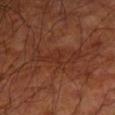Impression:
Part of a total-body skin-imaging series; this lesion was reviewed on a skin check and was not flagged for biopsy.
Image and clinical context:
The tile uses cross-polarized illumination. This image is a 15 mm lesion crop taken from a total-body photograph. The lesion is on the left upper arm. A male subject, approximately 65 years of age. Approximately 6 mm at its widest.